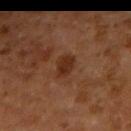Q: Was this lesion biopsied?
A: catalogued during a skin exam; not biopsied
Q: What is the lesion's diameter?
A: ≈3 mm
Q: Lesion location?
A: the right upper arm
Q: Who is the patient?
A: male, aged 58–62
Q: Automated lesion metrics?
A: a lesion area of about 4.5 mm², an outline eccentricity of about 0.8 (0 = round, 1 = elongated), and a symmetry-axis asymmetry near 0.25; an automated nevus-likeness rating near 45 out of 100 and a lesion-detection confidence of about 100/100
Q: What is the imaging modality?
A: total-body-photography crop, ~15 mm field of view